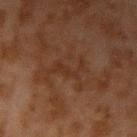Clinical impression: Captured during whole-body skin photography for melanoma surveillance; the lesion was not biopsied. Clinical summary: Automated tile analysis of the lesion measured an average lesion color of about L≈25 a*≈17 b*≈24 (CIELAB). The software also gave border irregularity of about 10 on a 0–10 scale, internal color variation of about 0.5 on a 0–10 scale, and radial color variation of about 0. The software also gave a classifier nevus-likeness of about 0/100 and a lesion-detection confidence of about 100/100. A male subject, aged around 45. Imaged with cross-polarized lighting. Cropped from a whole-body photographic skin survey; the tile spans about 15 mm. The lesion is located on the right upper arm.